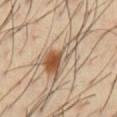<case>
  <biopsy_status>not biopsied; imaged during a skin examination</biopsy_status>
  <site>chest</site>
  <image>
    <source>total-body photography crop</source>
    <field_of_view_mm>15</field_of_view_mm>
  </image>
  <automated_metrics>
    <area_mm2_approx>12.0</area_mm2_approx>
    <eccentricity>0.9</eccentricity>
    <shape_asymmetry>0.55</shape_asymmetry>
    <cielab_L>54</cielab_L>
    <cielab_a>15</cielab_a>
    <cielab_b>31</cielab_b>
    <vs_skin_darker_L>12.0</vs_skin_darker_L>
    <vs_skin_contrast_norm>8.5</vs_skin_contrast_norm>
  </automated_metrics>
  <lesion_size>
    <long_diameter_mm_approx>6.0</long_diameter_mm_approx>
  </lesion_size>
  <lighting>cross-polarized</lighting>
  <patient>
    <sex>male</sex>
    <age_approx>40</age_approx>
  </patient>
</case>